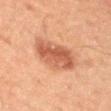Q: What is the anatomic site?
A: the right upper arm
Q: Patient demographics?
A: male, roughly 45 years of age
Q: What kind of image is this?
A: ~15 mm crop, total-body skin-cancer survey
Q: How was the tile lit?
A: cross-polarized
Q: Automated lesion metrics?
A: an outline eccentricity of about 0.2 (0 = round, 1 = elongated) and a symmetry-axis asymmetry near 0.15; a classifier nevus-likeness of about 85/100 and lesion-presence confidence of about 100/100
Q: How large is the lesion?
A: about 4 mm